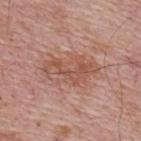Clinical impression:
Recorded during total-body skin imaging; not selected for excision or biopsy.
Context:
Cropped from a total-body skin-imaging series; the visible field is about 15 mm. On the upper back. About 6.5 mm across. A male patient, aged 63–67.